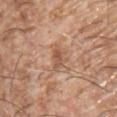follow-up — imaged on a skin check; not biopsied | location — the chest | image — ~15 mm crop, total-body skin-cancer survey | patient — male, aged around 65.